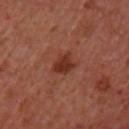The lesion was photographed on a routine skin check and not biopsied; there is no pathology result. The patient is a female roughly 40 years of age. The lesion is on the left upper arm. Imaged with cross-polarized lighting. The lesion's longest dimension is about 3 mm. A close-up tile cropped from a whole-body skin photograph, about 15 mm across.